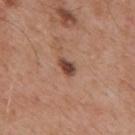No biopsy was performed on this lesion — it was imaged during a full skin examination and was not determined to be concerning. A 15 mm close-up tile from a total-body photography series done for melanoma screening. Automated image analysis of the tile measured a lesion area of about 3.5 mm², an eccentricity of roughly 0.75, and a symmetry-axis asymmetry near 0.3. It also reported a nevus-likeness score of about 90/100 and a detector confidence of about 100 out of 100 that the crop contains a lesion. From the mid back. A male subject aged approximately 55. The lesion's longest dimension is about 2.5 mm. The tile uses white-light illumination.